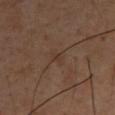<case>
<biopsy_status>not biopsied; imaged during a skin examination</biopsy_status>
<lighting>cross-polarized</lighting>
<lesion_size>
  <long_diameter_mm_approx>3.0</long_diameter_mm_approx>
</lesion_size>
<patient>
  <sex>male</sex>
  <age_approx>40</age_approx>
</patient>
<image>
  <source>total-body photography crop</source>
  <field_of_view_mm>15</field_of_view_mm>
</image>
<site>chest</site>
</case>